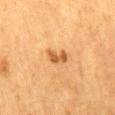Assessment: The lesion was photographed on a routine skin check and not biopsied; there is no pathology result. Image and clinical context: A 15 mm close-up tile from a total-body photography series done for melanoma screening. The subject is a female in their mid- to late 50s. Imaged with cross-polarized lighting. About 3 mm across. The lesion is on the mid back.